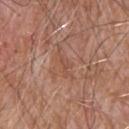<record>
<biopsy_status>not biopsied; imaged during a skin examination</biopsy_status>
<automated_metrics>
  <cielab_L>50</cielab_L>
  <cielab_a>22</cielab_a>
  <cielab_b>29</cielab_b>
  <vs_skin_darker_L>6.0</vs_skin_darker_L>
  <vs_skin_contrast_norm>4.5</vs_skin_contrast_norm>
  <border_irregularity_0_10>5.0</border_irregularity_0_10>
  <color_variation_0_10>0.0</color_variation_0_10>
</automated_metrics>
<patient>
  <sex>male</sex>
  <age_approx>80</age_approx>
</patient>
<lighting>white-light</lighting>
<lesion_size>
  <long_diameter_mm_approx>2.5</long_diameter_mm_approx>
</lesion_size>
<image>
  <source>total-body photography crop</source>
  <field_of_view_mm>15</field_of_view_mm>
</image>
<site>upper back</site>
</record>